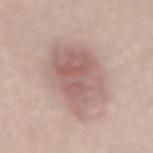This lesion was catalogued during total-body skin photography and was not selected for biopsy. A female subject, in their mid-60s. From the back. Longest diameter approximately 8 mm. Cropped from a total-body skin-imaging series; the visible field is about 15 mm. An algorithmic analysis of the crop reported an area of roughly 29 mm², an outline eccentricity of about 0.7 (0 = round, 1 = elongated), and a shape-asymmetry score of about 0.2 (0 = symmetric). It also reported border irregularity of about 2.5 on a 0–10 scale, a within-lesion color-variation index near 4.5/10, and radial color variation of about 1.5. The software also gave an automated nevus-likeness rating near 15 out of 100 and a detector confidence of about 100 out of 100 that the crop contains a lesion. Captured under white-light illumination.